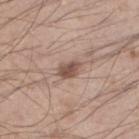{
  "lesion_size": {
    "long_diameter_mm_approx": 3.0
  },
  "lighting": "white-light",
  "automated_metrics": {
    "area_mm2_approx": 4.5,
    "eccentricity": 0.8,
    "shape_asymmetry": 0.25,
    "border_irregularity_0_10": 2.5,
    "color_variation_0_10": 2.5,
    "lesion_detection_confidence_0_100": 100
  },
  "image": {
    "source": "total-body photography crop",
    "field_of_view_mm": 15
  },
  "patient": {
    "sex": "male",
    "age_approx": 35
  },
  "site": "left lower leg"
}